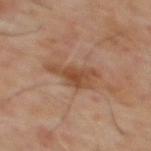Impression:
Imaged during a routine full-body skin examination; the lesion was not biopsied and no histopathology is available.
Acquisition and patient details:
A lesion tile, about 15 mm wide, cut from a 3D total-body photograph. The tile uses cross-polarized illumination. The lesion is located on the mid back. A male patient in their mid- to late 60s.